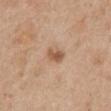| key | value |
|---|---|
| image | ~15 mm tile from a whole-body skin photo |
| patient | male, roughly 65 years of age |
| location | the mid back |